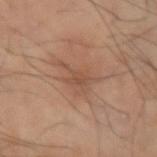Findings:
– follow-up: imaged on a skin check; not biopsied
– body site: the left forearm
– lesion diameter: ≈4.5 mm
– subject: male, in their mid- to late 60s
– lighting: cross-polarized illumination
– imaging modality: 15 mm crop, total-body photography
– automated metrics: a footprint of about 6 mm², an eccentricity of roughly 0.8, and two-axis asymmetry of about 0.4; a color-variation rating of about 2/10 and a peripheral color-asymmetry measure near 0.5; lesion-presence confidence of about 100/100